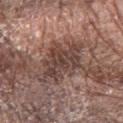This lesion was catalogued during total-body skin photography and was not selected for biopsy. A male subject, aged 68 to 72. The tile uses white-light illumination. A 15 mm crop from a total-body photograph taken for skin-cancer surveillance. From the left forearm.